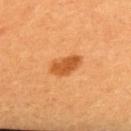biopsy status = catalogued during a skin exam; not biopsied.